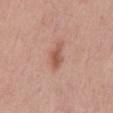The recorded lesion diameter is about 4 mm. Captured under white-light illumination. An algorithmic analysis of the crop reported an average lesion color of about L≈56 a*≈23 b*≈29 (CIELAB), roughly 10 lightness units darker than nearby skin, and a normalized lesion–skin contrast near 7. And it measured a border-irregularity rating of about 3/10, internal color variation of about 3 on a 0–10 scale, and peripheral color asymmetry of about 1. And it measured an automated nevus-likeness rating near 55 out of 100. A male subject aged approximately 60. A close-up tile cropped from a whole-body skin photograph, about 15 mm across. On the front of the torso.